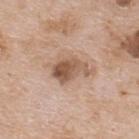Captured during whole-body skin photography for melanoma surveillance; the lesion was not biopsied. The patient is a male roughly 65 years of age. Located on the upper back. A lesion tile, about 15 mm wide, cut from a 3D total-body photograph. Approximately 4.5 mm at its widest. Automated image analysis of the tile measured an automated nevus-likeness rating near 30 out of 100 and a lesion-detection confidence of about 100/100.